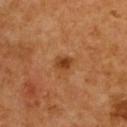The lesion was photographed on a routine skin check and not biopsied; there is no pathology result. Imaged with cross-polarized lighting. A 15 mm close-up extracted from a 3D total-body photography capture. The recorded lesion diameter is about 2 mm. A female subject in their mid-50s. From the upper back.